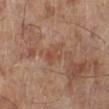notes = imaged on a skin check; not biopsied | image source = ~15 mm crop, total-body skin-cancer survey | anatomic site = the left lower leg | subject = male, aged approximately 70.